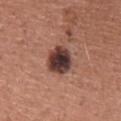| feature | finding |
|---|---|
| biopsy status | catalogued during a skin exam; not biopsied |
| location | the upper back |
| image source | ~15 mm crop, total-body skin-cancer survey |
| automated lesion analysis | a footprint of about 10 mm² and two-axis asymmetry of about 0.2; a peripheral color-asymmetry measure near 1.5; a classifier nevus-likeness of about 10/100 |
| patient | male, aged 43 to 47 |
| size | ~4 mm (longest diameter) |
| illumination | white-light illumination |